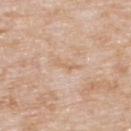{"biopsy_status": "not biopsied; imaged during a skin examination", "patient": {"sex": "male", "age_approx": 75}, "lesion_size": {"long_diameter_mm_approx": 2.5}, "automated_metrics": {"area_mm2_approx": 2.0, "eccentricity": 0.95, "shape_asymmetry": 0.45, "cielab_L": 65, "cielab_a": 18, "cielab_b": 34, "vs_skin_darker_L": 6.0, "vs_skin_contrast_norm": 5.0}, "image": {"source": "total-body photography crop", "field_of_view_mm": 15}, "site": "back", "lighting": "white-light"}